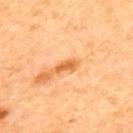Background: The lesion is on the upper back. Measured at roughly 2.5 mm in maximum diameter. A female subject, approximately 50 years of age. A close-up tile cropped from a whole-body skin photograph, about 15 mm across. The lesion-visualizer software estimated an eccentricity of roughly 0.9. This is a cross-polarized tile.